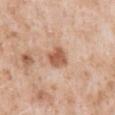workup: total-body-photography surveillance lesion; no biopsy | subject: male, approximately 50 years of age | lighting: white-light illumination | imaging modality: 15 mm crop, total-body photography | body site: the back.